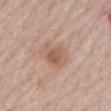Clinical impression:
The lesion was photographed on a routine skin check and not biopsied; there is no pathology result.
Acquisition and patient details:
Approximately 3.5 mm at its widest. An algorithmic analysis of the crop reported an area of roughly 8.5 mm² and two-axis asymmetry of about 0.3. It also reported border irregularity of about 3 on a 0–10 scale and a color-variation rating of about 3/10. It also reported a classifier nevus-likeness of about 10/100 and a lesion-detection confidence of about 100/100. On the back. A close-up tile cropped from a whole-body skin photograph, about 15 mm across. A female subject, aged 63 to 67.